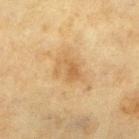{
  "biopsy_status": "not biopsied; imaged during a skin examination",
  "site": "left lower leg",
  "patient": {
    "sex": "female",
    "age_approx": 55
  },
  "image": {
    "source": "total-body photography crop",
    "field_of_view_mm": 15
  },
  "lesion_size": {
    "long_diameter_mm_approx": 4.0
  },
  "lighting": "cross-polarized"
}